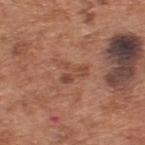Recorded during total-body skin imaging; not selected for excision or biopsy. Imaged with white-light lighting. From the upper back. A male subject about 65 years old. Automated image analysis of the tile measured an eccentricity of roughly 0.7 and two-axis asymmetry of about 0.65. And it measured a lesion-to-skin contrast of about 5.5 (normalized; higher = more distinct). The software also gave a nevus-likeness score of about 0/100 and a lesion-detection confidence of about 100/100. Approximately 3 mm at its widest. A 15 mm close-up tile from a total-body photography series done for melanoma screening.